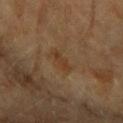follow-up — imaged on a skin check; not biopsied | site — the right forearm | illumination — cross-polarized | imaging modality — total-body-photography crop, ~15 mm field of view | lesion size — about 3 mm | automated metrics — peripheral color asymmetry of about 0 | subject — female, in their 60s.